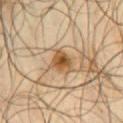Assessment:
Recorded during total-body skin imaging; not selected for excision or biopsy.
Image and clinical context:
A roughly 15 mm field-of-view crop from a total-body skin photograph. From the chest. A male subject, about 65 years old.